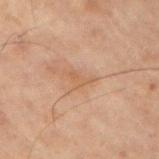follow-up: catalogued during a skin exam; not biopsied | tile lighting: cross-polarized illumination | image: total-body-photography crop, ~15 mm field of view | anatomic site: the right upper arm | lesion diameter: about 3 mm | patient: male, aged 68 to 72 | automated metrics: a footprint of about 3 mm² and a shape eccentricity near 0.85; an automated nevus-likeness rating near 0 out of 100 and a lesion-detection confidence of about 100/100.